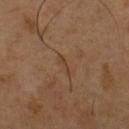This lesion was catalogued during total-body skin photography and was not selected for biopsy. A roughly 15 mm field-of-view crop from a total-body skin photograph. The subject is a male about 50 years old. From the chest.